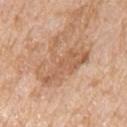Imaged during a routine full-body skin examination; the lesion was not biopsied and no histopathology is available. A roughly 15 mm field-of-view crop from a total-body skin photograph. A male patient roughly 65 years of age. Imaged with white-light lighting. Automated image analysis of the tile measured an area of roughly 18 mm² and an eccentricity of roughly 0.8. The software also gave a lesion color around L≈60 a*≈20 b*≈34 in CIELAB, about 9 CIELAB-L* units darker than the surrounding skin, and a normalized lesion–skin contrast near 6. And it measured a border-irregularity index near 8.5/10, a within-lesion color-variation index near 3/10, and a peripheral color-asymmetry measure near 1. And it measured a classifier nevus-likeness of about 0/100 and a lesion-detection confidence of about 95/100. Longest diameter approximately 7 mm. On the right upper arm.